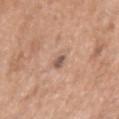The lesion is on the right upper arm.
Cropped from a total-body skin-imaging series; the visible field is about 15 mm.
The patient is a male aged approximately 65.